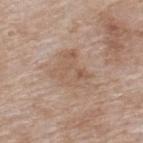notes: no biopsy performed (imaged during a skin exam) | lesion diameter: ≈5.5 mm | anatomic site: the upper back | subject: female, approximately 75 years of age | imaging modality: 15 mm crop, total-body photography | image-analysis metrics: a shape eccentricity near 0.7 and two-axis asymmetry of about 0.45; a mean CIELAB color near L≈57 a*≈16 b*≈28 and a lesion–skin lightness drop of about 7 | tile lighting: white-light illumination.